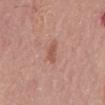Assessment: Imaged during a routine full-body skin examination; the lesion was not biopsied and no histopathology is available. Background: On the mid back. Approximately 2.5 mm at its widest. Captured under white-light illumination. The patient is a male aged 63 to 67. A lesion tile, about 15 mm wide, cut from a 3D total-body photograph.